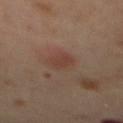Cropped from a whole-body photographic skin survey; the tile spans about 15 mm. About 3 mm across. Located on the mid back. A female subject about 65 years old. Captured under cross-polarized illumination.